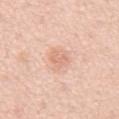notes: no biopsy performed (imaged during a skin exam)
illumination: white-light
acquisition: ~15 mm tile from a whole-body skin photo
TBP lesion metrics: an area of roughly 6 mm², an eccentricity of roughly 0.7, and a shape-asymmetry score of about 0.35 (0 = symmetric); an average lesion color of about L≈71 a*≈22 b*≈31 (CIELAB) and roughly 8 lightness units darker than nearby skin; a border-irregularity rating of about 3.5/10, a color-variation rating of about 2/10, and a peripheral color-asymmetry measure near 0.5; a nevus-likeness score of about 0/100 and a detector confidence of about 100 out of 100 that the crop contains a lesion
subject: male, in their mid-50s
anatomic site: the abdomen
size: ~3.5 mm (longest diameter)